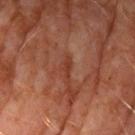Notes:
- workup: no biopsy performed (imaged during a skin exam)